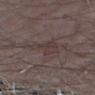• workup · total-body-photography surveillance lesion; no biopsy
• subject · male, aged 63–67
• site · the arm
• lighting · white-light
• TBP lesion metrics · a footprint of about 6 mm² and an eccentricity of roughly 0.4
• imaging modality · total-body-photography crop, ~15 mm field of view